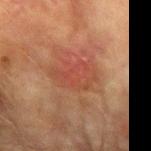Q: Was a biopsy performed?
A: catalogued during a skin exam; not biopsied
Q: Automated lesion metrics?
A: a border-irregularity index near 3/10 and radial color variation of about 1
Q: What are the patient's age and sex?
A: male, roughly 75 years of age
Q: What lighting was used for the tile?
A: cross-polarized illumination
Q: What is the imaging modality?
A: 15 mm crop, total-body photography
Q: What is the anatomic site?
A: the right forearm
Q: What is the lesion's diameter?
A: ~4.5 mm (longest diameter)